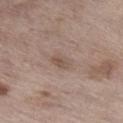{"biopsy_status": "not biopsied; imaged during a skin examination", "site": "leg", "lesion_size": {"long_diameter_mm_approx": 2.5}, "image": {"source": "total-body photography crop", "field_of_view_mm": 15}, "patient": {"sex": "male", "age_approx": 70}, "lighting": "white-light"}